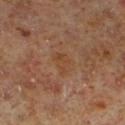Notes:
* notes · no biopsy performed (imaged during a skin exam)
* automated metrics · a classifier nevus-likeness of about 0/100 and a detector confidence of about 100 out of 100 that the crop contains a lesion
* lighting · cross-polarized illumination
* body site · the left lower leg
* acquisition · ~15 mm crop, total-body skin-cancer survey
* subject · male, aged 58–62